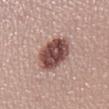biopsy status — imaged on a skin check; not biopsied
anatomic site — the right lower leg
lesion diameter — about 4.5 mm
acquisition — ~15 mm tile from a whole-body skin photo
subject — female, aged approximately 25